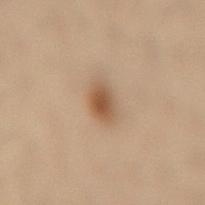{"patient": {"sex": "female", "age_approx": 55}, "site": "lower back", "lesion_size": {"long_diameter_mm_approx": 3.0}, "automated_metrics": {"area_mm2_approx": 5.5, "eccentricity": 0.65, "shape_asymmetry": 0.2, "cielab_L": 44, "cielab_a": 15, "cielab_b": 28, "vs_skin_darker_L": 10.0, "vs_skin_contrast_norm": 8.0}, "image": {"source": "total-body photography crop", "field_of_view_mm": 15}, "lighting": "cross-polarized"}